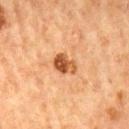Assessment: This lesion was catalogued during total-body skin photography and was not selected for biopsy. Acquisition and patient details: The lesion's longest dimension is about 3 mm. A 15 mm close-up extracted from a 3D total-body photography capture. The subject is a male in their mid- to late 60s. An algorithmic analysis of the crop reported a lesion color around L≈48 a*≈25 b*≈37 in CIELAB, about 14 CIELAB-L* units darker than the surrounding skin, and a normalized lesion–skin contrast near 10. And it measured an automated nevus-likeness rating near 95 out of 100. Imaged with cross-polarized lighting. On the back.